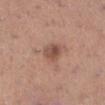workup = total-body-photography surveillance lesion; no biopsy
acquisition = ~15 mm crop, total-body skin-cancer survey
tile lighting = white-light
size = ~2.5 mm (longest diameter)
TBP lesion metrics = an eccentricity of roughly 0.6 and two-axis asymmetry of about 0.2; a mean CIELAB color near L≈50 a*≈21 b*≈27 and a normalized border contrast of about 7.5; an automated nevus-likeness rating near 85 out of 100
subject = female, aged 28–32
site = the right lower leg